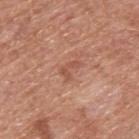The lesion was photographed on a routine skin check and not biopsied; there is no pathology result. A male subject, aged 63 to 67. Located on the upper back. A 15 mm crop from a total-body photograph taken for skin-cancer surveillance.